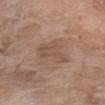| key | value |
|---|---|
| follow-up | imaged on a skin check; not biopsied |
| image source | ~15 mm tile from a whole-body skin photo |
| body site | the arm |
| diameter | about 4 mm |
| image-analysis metrics | a footprint of about 9 mm², an eccentricity of roughly 0.55, and a shape-asymmetry score of about 0.45 (0 = symmetric) |
| tile lighting | white-light illumination |
| patient | female, roughly 75 years of age |